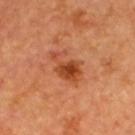biopsy status: imaged on a skin check; not biopsied | subject: male, aged 63 to 67 | imaging modality: 15 mm crop, total-body photography | site: the back | lesion diameter: about 4.5 mm | illumination: cross-polarized illumination | automated lesion analysis: a lesion area of about 8 mm², an outline eccentricity of about 0.8 (0 = round, 1 = elongated), and a symmetry-axis asymmetry near 0.4; an automated nevus-likeness rating near 90 out of 100 and a lesion-detection confidence of about 100/100.